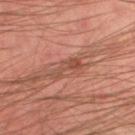workup: no biopsy performed (imaged during a skin exam)
subject: male, aged around 45
imaging modality: ~15 mm crop, total-body skin-cancer survey
location: the left thigh
lesion diameter: ~4 mm (longest diameter)
image-analysis metrics: a lesion area of about 4.5 mm², an eccentricity of roughly 0.9, and two-axis asymmetry of about 0.45